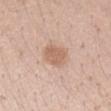No biopsy was performed on this lesion — it was imaged during a full skin examination and was not determined to be concerning.
The lesion is located on the right forearm.
The tile uses white-light illumination.
The subject is a female approximately 20 years of age.
Measured at roughly 3 mm in maximum diameter.
An algorithmic analysis of the crop reported an eccentricity of roughly 0.65 and a symmetry-axis asymmetry near 0.2. The analysis additionally found an average lesion color of about L≈62 a*≈20 b*≈30 (CIELAB) and a normalized border contrast of about 6.5. And it measured a border-irregularity index near 2/10, a within-lesion color-variation index near 2/10, and a peripheral color-asymmetry measure near 0.5. It also reported an automated nevus-likeness rating near 70 out of 100 and a detector confidence of about 100 out of 100 that the crop contains a lesion.
A region of skin cropped from a whole-body photographic capture, roughly 15 mm wide.